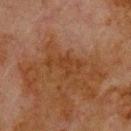follow-up: no biopsy performed (imaged during a skin exam)
site: the upper back
subject: male, aged around 80
acquisition: ~15 mm tile from a whole-body skin photo
size: about 4.5 mm
lighting: cross-polarized illumination
TBP lesion metrics: a mean CIELAB color near L≈31 a*≈18 b*≈29, roughly 5 lightness units darker than nearby skin, and a normalized lesion–skin contrast near 5.5